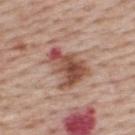workup=total-body-photography surveillance lesion; no biopsy